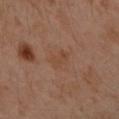Imaged during a routine full-body skin examination; the lesion was not biopsied and no histopathology is available.
A female patient, aged 38 to 42.
From the left forearm.
This image is a 15 mm lesion crop taken from a total-body photograph.